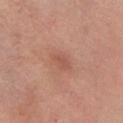notes = total-body-photography surveillance lesion; no biopsy